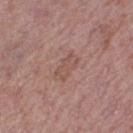Assessment: This lesion was catalogued during total-body skin photography and was not selected for biopsy. Clinical summary: Located on the leg. A 15 mm crop from a total-body photograph taken for skin-cancer surveillance. The recorded lesion diameter is about 3.5 mm. A female subject aged 48–52. Imaged with white-light lighting. Automated image analysis of the tile measured a footprint of about 6 mm², an outline eccentricity of about 0.85 (0 = round, 1 = elongated), and a symmetry-axis asymmetry near 0.25. It also reported a classifier nevus-likeness of about 0/100 and a lesion-detection confidence of about 100/100.